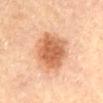biopsy status: imaged on a skin check; not biopsied
image source: 15 mm crop, total-body photography
location: the back
subject: male, aged around 85 or older